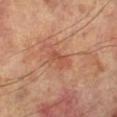Q: Was a biopsy performed?
A: imaged on a skin check; not biopsied
Q: What is the lesion's diameter?
A: ≈2.5 mm
Q: Automated lesion metrics?
A: an area of roughly 3 mm², a shape eccentricity near 0.85, and two-axis asymmetry of about 0.25; a border-irregularity index near 2.5/10 and radial color variation of about 0.5
Q: What lighting was used for the tile?
A: cross-polarized
Q: What kind of image is this?
A: ~15 mm crop, total-body skin-cancer survey
Q: Who is the patient?
A: male, aged 68–72
Q: Lesion location?
A: the left lower leg